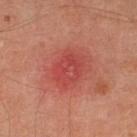The lesion was photographed on a routine skin check and not biopsied; there is no pathology result. The recorded lesion diameter is about 4 mm. A region of skin cropped from a whole-body photographic capture, roughly 15 mm wide. From the left lower leg. Captured under cross-polarized illumination. The subject is about 55 years old.